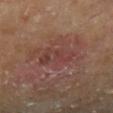This is a cross-polarized tile.
Approximately 4 mm at its widest.
The lesion is on the left lower leg.
The lesion-visualizer software estimated a lesion color around L≈39 a*≈22 b*≈23 in CIELAB, about 5 CIELAB-L* units darker than the surrounding skin, and a lesion-to-skin contrast of about 5 (normalized; higher = more distinct). The analysis additionally found border irregularity of about 3.5 on a 0–10 scale, a within-lesion color-variation index near 6.5/10, and radial color variation of about 2.5.
A male subject about 65 years old.
A 15 mm crop from a total-body photograph taken for skin-cancer surveillance.